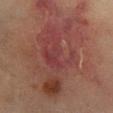• follow-up · catalogued during a skin exam; not biopsied
• image · ~15 mm crop, total-body skin-cancer survey
• diameter · about 10 mm
• subject · female, roughly 70 years of age
• tile lighting · cross-polarized
• anatomic site · the right lower leg